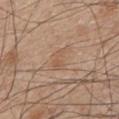Imaged during a routine full-body skin examination; the lesion was not biopsied and no histopathology is available.
Longest diameter approximately 4 mm.
Located on the mid back.
A 15 mm crop from a total-body photograph taken for skin-cancer surveillance.
This is a white-light tile.
A male subject aged 63–67.